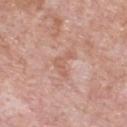This lesion was catalogued during total-body skin photography and was not selected for biopsy.
A close-up tile cropped from a whole-body skin photograph, about 15 mm across.
About 4 mm across.
Located on the chest.
A male patient in their mid- to late 50s.
An algorithmic analysis of the crop reported an area of roughly 6 mm², a shape eccentricity near 0.75, and a shape-asymmetry score of about 0.7 (0 = symmetric). And it measured lesion-presence confidence of about 100/100.